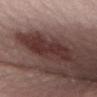Part of a total-body skin-imaging series; this lesion was reviewed on a skin check and was not flagged for biopsy.
The tile uses white-light illumination.
A region of skin cropped from a whole-body photographic capture, roughly 15 mm wide.
On the lower back.
The lesion's longest dimension is about 6 mm.
The total-body-photography lesion software estimated an outline eccentricity of about 0.9 (0 = round, 1 = elongated) and a shape-asymmetry score of about 0.25 (0 = symmetric). The software also gave a mean CIELAB color near L≈32 a*≈20 b*≈19 and a normalized lesion–skin contrast near 9. The analysis additionally found border irregularity of about 4 on a 0–10 scale, a within-lesion color-variation index near 3/10, and a peripheral color-asymmetry measure near 1.
The subject is a female aged around 40.